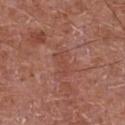Clinical impression:
No biopsy was performed on this lesion — it was imaged during a full skin examination and was not determined to be concerning.